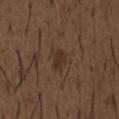Clinical impression: Captured during whole-body skin photography for melanoma surveillance; the lesion was not biopsied. Background: The lesion is on the chest. Imaged with white-light lighting. The lesion's longest dimension is about 3 mm. A male patient, aged 48 to 52. A 15 mm close-up tile from a total-body photography series done for melanoma screening.